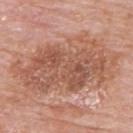follow-up: total-body-photography surveillance lesion; no biopsy | image source: total-body-photography crop, ~15 mm field of view | tile lighting: white-light | subject: male, roughly 80 years of age | site: the upper back.